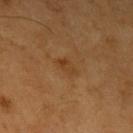follow-up: catalogued during a skin exam; not biopsied | automated lesion analysis: a footprint of about 3.5 mm² and an outline eccentricity of about 0.8 (0 = round, 1 = elongated); a lesion color around L≈39 a*≈20 b*≈36 in CIELAB and a lesion–skin lightness drop of about 6; a lesion-detection confidence of about 100/100 | image source: 15 mm crop, total-body photography | anatomic site: the left upper arm | illumination: cross-polarized | patient: male, roughly 60 years of age | lesion diameter: about 2.5 mm.